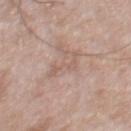- biopsy status: catalogued during a skin exam; not biopsied
- subject: male, in their 70s
- lesion diameter: about 4.5 mm
- site: the front of the torso
- automated metrics: a detector confidence of about 65 out of 100 that the crop contains a lesion
- image source: ~15 mm tile from a whole-body skin photo